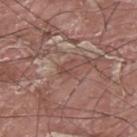Q: Was a biopsy performed?
A: catalogued during a skin exam; not biopsied
Q: What is the imaging modality?
A: ~15 mm tile from a whole-body skin photo
Q: Where on the body is the lesion?
A: the upper back
Q: Who is the patient?
A: male, about 40 years old